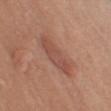workup: catalogued during a skin exam; not biopsied
site: the arm
size: about 5.5 mm
subject: male, in their mid-70s
imaging modality: 15 mm crop, total-body photography